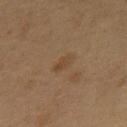No biopsy was performed on this lesion — it was imaged during a full skin examination and was not determined to be concerning.
Imaged with cross-polarized lighting.
Automated image analysis of the tile measured a footprint of about 4.5 mm², an eccentricity of roughly 0.85, and two-axis asymmetry of about 0.2. The analysis additionally found border irregularity of about 2 on a 0–10 scale and a within-lesion color-variation index near 2/10.
A male patient, in their mid- to late 50s.
A 15 mm close-up tile from a total-body photography series done for melanoma screening.
Measured at roughly 3 mm in maximum diameter.
Located on the mid back.